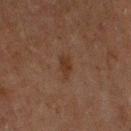workup: imaged on a skin check; not biopsied | imaging modality: ~15 mm crop, total-body skin-cancer survey | lighting: cross-polarized | location: the right upper arm | image-analysis metrics: a footprint of about 3 mm², an eccentricity of roughly 0.9, and two-axis asymmetry of about 0.35; an automated nevus-likeness rating near 15 out of 100 | subject: male, aged 58 to 62 | size: about 3 mm.